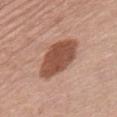Part of a total-body skin-imaging series; this lesion was reviewed on a skin check and was not flagged for biopsy. About 6 mm across. The subject is a female aged around 60. The lesion is on the abdomen. A 15 mm close-up tile from a total-body photography series done for melanoma screening.